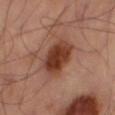From the right thigh. Captured under cross-polarized illumination. A roughly 15 mm field-of-view crop from a total-body skin photograph. Automated tile analysis of the lesion measured two-axis asymmetry of about 0.15. It also reported a mean CIELAB color near L≈40 a*≈24 b*≈30 and about 14 CIELAB-L* units darker than the surrounding skin. The analysis additionally found an automated nevus-likeness rating near 95 out of 100.